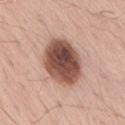workup — catalogued during a skin exam; not biopsied
image source — 15 mm crop, total-body photography
location — the back
automated lesion analysis — an automated nevus-likeness rating near 30 out of 100 and lesion-presence confidence of about 100/100
illumination — white-light
subject — male, about 55 years old